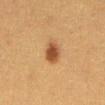* biopsy status — imaged on a skin check; not biopsied
* body site — the abdomen
* lesion diameter — ≈2.5 mm
* image — 15 mm crop, total-body photography
* subject — female, roughly 40 years of age